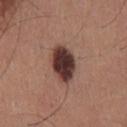acquisition: ~15 mm tile from a whole-body skin photo | patient: male, in their 40s | location: the mid back | automated lesion analysis: a lesion color around L≈36 a*≈20 b*≈21 in CIELAB and a lesion-to-skin contrast of about 15 (normalized; higher = more distinct); an automated nevus-likeness rating near 60 out of 100 and a detector confidence of about 100 out of 100 that the crop contains a lesion | size: ~5 mm (longest diameter).